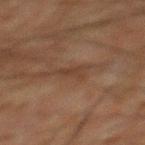Impression:
Captured during whole-body skin photography for melanoma surveillance; the lesion was not biopsied.
Acquisition and patient details:
From the mid back. Automated tile analysis of the lesion measured a lesion area of about 2.5 mm², a shape eccentricity near 0.85, and a shape-asymmetry score of about 0.45 (0 = symmetric). The software also gave a border-irregularity index near 5/10 and a color-variation rating of about 0/10. A male subject aged approximately 65. A 15 mm close-up extracted from a 3D total-body photography capture. The lesion's longest dimension is about 2.5 mm. This is a cross-polarized tile.